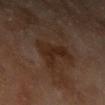Clinical impression:
The lesion was tiled from a total-body skin photograph and was not biopsied.
Clinical summary:
The subject is a male aged around 70. The lesion is located on the left upper arm. A roughly 15 mm field-of-view crop from a total-body skin photograph.